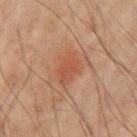Context: On the left upper arm. Measured at roughly 4.5 mm in maximum diameter. A male patient in their mid-60s. This image is a 15 mm lesion crop taken from a total-body photograph. This is a cross-polarized tile.